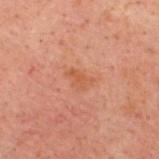| key | value |
|---|---|
| imaging modality | ~15 mm tile from a whole-body skin photo |
| subject | male, approximately 40 years of age |
| size | about 3 mm |
| tile lighting | cross-polarized |
| image-analysis metrics | a footprint of about 4 mm² and a symmetry-axis asymmetry near 0.4; a color-variation rating of about 1.5/10 and a peripheral color-asymmetry measure near 0.5 |
| site | the upper back |